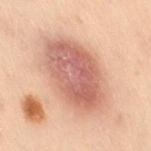workup = imaged on a skin check; not biopsied
imaging modality = ~15 mm crop, total-body skin-cancer survey
patient = female, about 50 years old
automated lesion analysis = an average lesion color of about L≈53 a*≈21 b*≈25 (CIELAB); internal color variation of about 5 on a 0–10 scale and a peripheral color-asymmetry measure near 1.5; a classifier nevus-likeness of about 100/100 and a lesion-detection confidence of about 100/100
location = the right thigh
size = ≈8 mm
tile lighting = cross-polarized illumination